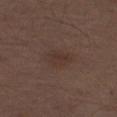The lesion was tiled from a total-body skin photograph and was not biopsied. A 15 mm crop from a total-body photograph taken for skin-cancer surveillance. About 3.5 mm across. The lesion is located on the left thigh. A male subject aged 68–72. Imaged with white-light lighting.